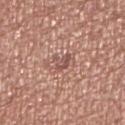Case summary:
– notes: imaged on a skin check; not biopsied
– patient: male, approximately 70 years of age
– lighting: white-light illumination
– image-analysis metrics: a classifier nevus-likeness of about 0/100 and a lesion-detection confidence of about 65/100
– acquisition: total-body-photography crop, ~15 mm field of view
– lesion size: ≈3 mm
– location: the right lower leg